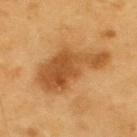Impression:
Part of a total-body skin-imaging series; this lesion was reviewed on a skin check and was not flagged for biopsy.
Background:
The subject is a male roughly 60 years of age. The lesion is on the back. Captured under cross-polarized illumination. Longest diameter approximately 8 mm. A lesion tile, about 15 mm wide, cut from a 3D total-body photograph.